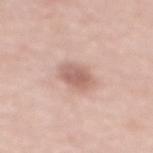{"biopsy_status": "not biopsied; imaged during a skin examination", "lesion_size": {"long_diameter_mm_approx": 3.5}, "image": {"source": "total-body photography crop", "field_of_view_mm": 15}, "site": "chest", "automated_metrics": {"border_irregularity_0_10": 1.5, "color_variation_0_10": 3.5}, "patient": {"sex": "female", "age_approx": 65}}